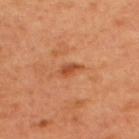Clinical summary:
A roughly 15 mm field-of-view crop from a total-body skin photograph. Imaged with cross-polarized lighting. From the upper back. The lesion's longest dimension is about 2.5 mm. A male patient, in their 50s. The lesion-visualizer software estimated a footprint of about 3 mm², a shape eccentricity near 0.85, and a shape-asymmetry score of about 0.3 (0 = symmetric).